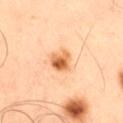{
  "site": "leg",
  "automated_metrics": {
    "eccentricity": 0.6,
    "shape_asymmetry": 0.2,
    "cielab_L": 66,
    "cielab_a": 27,
    "cielab_b": 44,
    "vs_skin_darker_L": 15.0,
    "vs_skin_contrast_norm": 9.5,
    "color_variation_0_10": 8.5,
    "nevus_likeness_0_100": 95,
    "lesion_detection_confidence_0_100": 100
  },
  "patient": {
    "sex": "male",
    "age_approx": 50
  },
  "lesion_size": {
    "long_diameter_mm_approx": 3.0
  },
  "image": {
    "source": "total-body photography crop",
    "field_of_view_mm": 15
  }
}